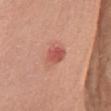Q: Was a biopsy performed?
A: catalogued during a skin exam; not biopsied
Q: How large is the lesion?
A: ~3 mm (longest diameter)
Q: Who is the patient?
A: female, approximately 60 years of age
Q: Where on the body is the lesion?
A: the left upper arm
Q: How was the tile lit?
A: white-light
Q: What kind of image is this?
A: ~15 mm tile from a whole-body skin photo